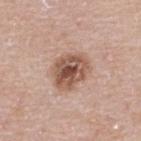The lesion was photographed on a routine skin check and not biopsied; there is no pathology result. A female patient aged approximately 40. A 15 mm close-up tile from a total-body photography series done for melanoma screening. On the upper back. The lesion's longest dimension is about 5 mm. Imaged with white-light lighting.